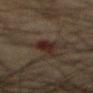Background:
A male subject aged approximately 65. Located on the abdomen. Measured at roughly 3.5 mm in maximum diameter. The tile uses cross-polarized illumination. A region of skin cropped from a whole-body photographic capture, roughly 15 mm wide. The total-body-photography lesion software estimated a nevus-likeness score of about 15/100.